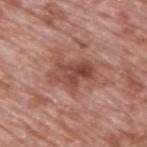Impression: This lesion was catalogued during total-body skin photography and was not selected for biopsy. Background: The lesion is on the upper back. A male subject, aged around 70. A region of skin cropped from a whole-body photographic capture, roughly 15 mm wide.